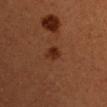<tbp_lesion>
  <image>
    <source>total-body photography crop</source>
    <field_of_view_mm>15</field_of_view_mm>
  </image>
  <patient>
    <sex>female</sex>
    <age_approx>50</age_approx>
  </patient>
  <lighting>cross-polarized</lighting>
  <site>right upper arm</site>
  <lesion_size>
    <long_diameter_mm_approx>2.5</long_diameter_mm_approx>
  </lesion_size>
  <diagnosis>
    <histopathology>atypical melanocytic neoplasm</histopathology>
    <malignancy>indeterminate</malignancy>
    <taxonomic_path>Indeterminate, Indeterminate melanocytic proliferations, Atypical melanocytic neoplasm</taxonomic_path>
  </diagnosis>
</tbp_lesion>